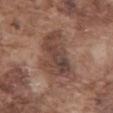The lesion was tiled from a total-body skin photograph and was not biopsied.